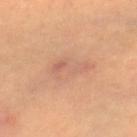Recorded during total-body skin imaging; not selected for excision or biopsy. A female patient aged 43–47. About 4.5 mm across. From the leg. Cropped from a whole-body photographic skin survey; the tile spans about 15 mm.